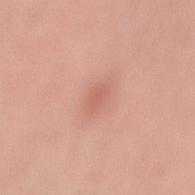Recorded during total-body skin imaging; not selected for excision or biopsy. The subject is a male approximately 45 years of age. A lesion tile, about 15 mm wide, cut from a 3D total-body photograph. Captured under white-light illumination. The lesion's longest dimension is about 3 mm. The lesion is on the lower back.